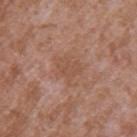Captured during whole-body skin photography for melanoma surveillance; the lesion was not biopsied. This is a white-light tile. Located on the left upper arm. A 15 mm close-up tile from a total-body photography series done for melanoma screening. The total-body-photography lesion software estimated a lesion area of about 7.5 mm², a shape eccentricity near 0.35, and a symmetry-axis asymmetry near 0.35. The software also gave border irregularity of about 4 on a 0–10 scale and radial color variation of about 1. It also reported a classifier nevus-likeness of about 0/100. The lesion's longest dimension is about 3.5 mm. A male patient about 45 years old.